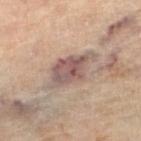<tbp_lesion>
  <biopsy_status>not biopsied; imaged during a skin examination</biopsy_status>
  <patient>
    <sex>female</sex>
    <age_approx>60</age_approx>
  </patient>
  <site>right lower leg</site>
  <image>
    <source>total-body photography crop</source>
    <field_of_view_mm>15</field_of_view_mm>
  </image>
</tbp_lesion>